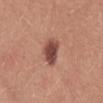Findings:
– follow-up — total-body-photography surveillance lesion; no biopsy
– lesion size — about 3.5 mm
– illumination — white-light
– patient — female, aged 23 to 27
– image source — ~15 mm tile from a whole-body skin photo
– location — the chest